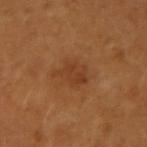notes — imaged on a skin check; not biopsied
lighting — cross-polarized
location — the right upper arm
imaging modality — 15 mm crop, total-body photography
TBP lesion metrics — an outline eccentricity of about 0.6 (0 = round, 1 = elongated) and two-axis asymmetry of about 0.25; an average lesion color of about L≈41 a*≈23 b*≈36 (CIELAB), a lesion–skin lightness drop of about 6, and a normalized border contrast of about 5.5; a border-irregularity index near 3/10, a within-lesion color-variation index near 2.5/10, and radial color variation of about 1
size — about 4 mm
patient — female, roughly 55 years of age